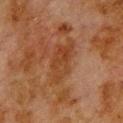• notes · total-body-photography surveillance lesion; no biopsy
• imaging modality · 15 mm crop, total-body photography
• site · the upper back
• image-analysis metrics · a lesion color around L≈32 a*≈19 b*≈29 in CIELAB, roughly 6 lightness units darker than nearby skin, and a normalized border contrast of about 6.5; a classifier nevus-likeness of about 0/100 and a lesion-detection confidence of about 100/100
• subject · male, aged 78 to 82
• illumination · cross-polarized
• lesion diameter · ≈5.5 mm